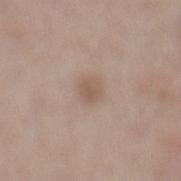Assessment: The lesion was tiled from a total-body skin photograph and was not biopsied. Background: A male subject, aged approximately 70. A close-up tile cropped from a whole-body skin photograph, about 15 mm across. Imaged with white-light lighting. The recorded lesion diameter is about 3 mm. From the back.